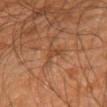Clinical impression:
This lesion was catalogued during total-body skin photography and was not selected for biopsy.
Background:
A male subject, aged approximately 65. From the right upper arm. Cropped from a total-body skin-imaging series; the visible field is about 15 mm.